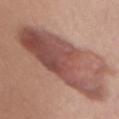Cropped from a whole-body photographic skin survey; the tile spans about 15 mm.
Imaged with white-light lighting.
The total-body-photography lesion software estimated border irregularity of about 6 on a 0–10 scale, a color-variation rating of about 7.5/10, and a peripheral color-asymmetry measure near 2.5. The software also gave an automated nevus-likeness rating near 10 out of 100.
The recorded lesion diameter is about 12 mm.
The subject is a female aged approximately 45.
Located on the chest.